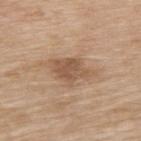Q: Was a biopsy performed?
A: total-body-photography surveillance lesion; no biopsy
Q: What is the lesion's diameter?
A: ≈5 mm
Q: How was the tile lit?
A: white-light illumination
Q: How was this image acquired?
A: ~15 mm crop, total-body skin-cancer survey
Q: What is the anatomic site?
A: the upper back
Q: What are the patient's age and sex?
A: female, in their mid- to late 70s